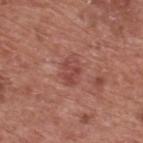Imaged during a routine full-body skin examination; the lesion was not biopsied and no histopathology is available.